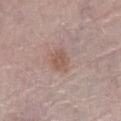Impression:
Imaged during a routine full-body skin examination; the lesion was not biopsied and no histopathology is available.
Image and clinical context:
A male patient aged 78–82. Captured under white-light illumination. Cropped from a total-body skin-imaging series; the visible field is about 15 mm. The lesion-visualizer software estimated a footprint of about 5 mm² and a shape eccentricity near 0.55. It also reported a lesion color around L≈55 a*≈18 b*≈25 in CIELAB and a lesion-to-skin contrast of about 6.5 (normalized; higher = more distinct). The software also gave a border-irregularity index near 2/10, internal color variation of about 1.5 on a 0–10 scale, and peripheral color asymmetry of about 0.5. On the right lower leg. The lesion's longest dimension is about 2.5 mm.